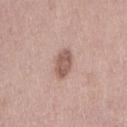Imaged during a routine full-body skin examination; the lesion was not biopsied and no histopathology is available. Imaged with white-light lighting. Longest diameter approximately 3.5 mm. A close-up tile cropped from a whole-body skin photograph, about 15 mm across. From the right thigh. A female patient aged 38–42.